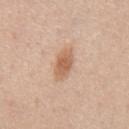Case summary:
• notes — imaged on a skin check; not biopsied
• acquisition — ~15 mm crop, total-body skin-cancer survey
• lighting — white-light illumination
• patient — male, aged approximately 60
• lesion size — ~4 mm (longest diameter)
• location — the mid back